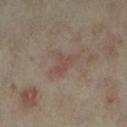Clinical impression:
The lesion was photographed on a routine skin check and not biopsied; there is no pathology result.
Clinical summary:
The subject is a female aged 33–37. Cropped from a total-body skin-imaging series; the visible field is about 15 mm. The tile uses cross-polarized illumination. Automated image analysis of the tile measured a within-lesion color-variation index near 1.5/10 and a peripheral color-asymmetry measure near 0.5. The analysis additionally found lesion-presence confidence of about 100/100. Approximately 3.5 mm at its widest. The lesion is located on the leg.